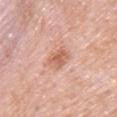| feature | finding |
|---|---|
| biopsy status | catalogued during a skin exam; not biopsied |
| site | the chest |
| subject | male, in their mid-50s |
| illumination | white-light illumination |
| imaging modality | 15 mm crop, total-body photography |
| size | ≈3.5 mm |